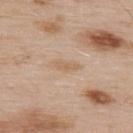Recorded during total-body skin imaging; not selected for excision or biopsy.
A male patient, about 55 years old.
The lesion-visualizer software estimated an area of roughly 3.5 mm², a shape eccentricity near 0.9, and a symmetry-axis asymmetry near 0.3. The analysis additionally found a normalized border contrast of about 5.5. It also reported a border-irregularity index near 3.5/10 and internal color variation of about 0.5 on a 0–10 scale.
A close-up tile cropped from a whole-body skin photograph, about 15 mm across.
This is a white-light tile.
Located on the upper back.